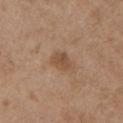workup: no biopsy performed (imaged during a skin exam)
patient: female, approximately 65 years of age
site: the chest
image-analysis metrics: a mean CIELAB color near L≈50 a*≈18 b*≈31, roughly 8 lightness units darker than nearby skin, and a lesion-to-skin contrast of about 6.5 (normalized; higher = more distinct); a border-irregularity rating of about 2/10, a color-variation rating of about 2/10, and a peripheral color-asymmetry measure near 0.5
imaging modality: ~15 mm tile from a whole-body skin photo
lesion diameter: about 3 mm